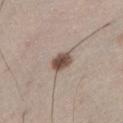workup: imaged on a skin check; not biopsied | diameter: about 3 mm | TBP lesion metrics: a footprint of about 5 mm², a shape eccentricity near 0.7, and a symmetry-axis asymmetry near 0.1; an automated nevus-likeness rating near 95 out of 100 and a detector confidence of about 100 out of 100 that the crop contains a lesion | lighting: white-light illumination | acquisition: ~15 mm crop, total-body skin-cancer survey | anatomic site: the left lower leg | patient: male, aged approximately 45.